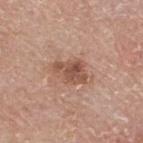Q: Was this lesion biopsied?
A: catalogued during a skin exam; not biopsied